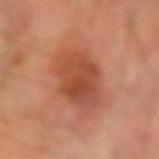This lesion was catalogued during total-body skin photography and was not selected for biopsy. Automated image analysis of the tile measured radial color variation of about 2. And it measured an automated nevus-likeness rating near 45 out of 100 and lesion-presence confidence of about 100/100. A male subject approximately 70 years of age. A 15 mm close-up tile from a total-body photography series done for melanoma screening. Located on the right forearm. This is a cross-polarized tile.